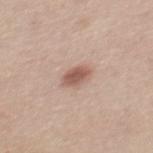Recorded during total-body skin imaging; not selected for excision or biopsy.